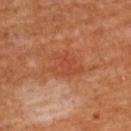Impression:
The lesion was tiled from a total-body skin photograph and was not biopsied.
Acquisition and patient details:
A close-up tile cropped from a whole-body skin photograph, about 15 mm across. A female patient, aged approximately 65. The lesion is located on the upper back. This is a cross-polarized tile.